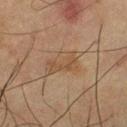notes = total-body-photography surveillance lesion; no biopsy | image source = ~15 mm crop, total-body skin-cancer survey | subject = male, roughly 65 years of age | tile lighting = cross-polarized illumination | anatomic site = the left thigh.